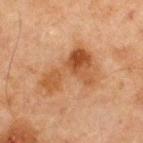Impression:
Recorded during total-body skin imaging; not selected for excision or biopsy.
Clinical summary:
Automated image analysis of the tile measured a lesion area of about 18 mm² and a shape eccentricity near 0.85. It also reported a mean CIELAB color near L≈42 a*≈20 b*≈32, about 9 CIELAB-L* units darker than the surrounding skin, and a normalized border contrast of about 8. The analysis additionally found a border-irregularity index near 7/10, a within-lesion color-variation index near 6.5/10, and radial color variation of about 2.5. The analysis additionally found a detector confidence of about 100 out of 100 that the crop contains a lesion. From the chest. A close-up tile cropped from a whole-body skin photograph, about 15 mm across. A male subject aged around 65.